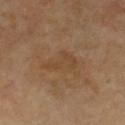Captured during whole-body skin photography for melanoma surveillance; the lesion was not biopsied. A male subject aged 63–67. The recorded lesion diameter is about 4.5 mm. The tile uses cross-polarized illumination. Automated tile analysis of the lesion measured an area of roughly 6 mm², an outline eccentricity of about 0.9 (0 = round, 1 = elongated), and two-axis asymmetry of about 0.6. It also reported a lesion color around L≈42 a*≈16 b*≈31 in CIELAB, a lesion–skin lightness drop of about 5, and a normalized border contrast of about 4.5. And it measured a border-irregularity rating of about 7/10 and peripheral color asymmetry of about 0.5. The lesion is located on the chest. A 15 mm crop from a total-body photograph taken for skin-cancer surveillance.